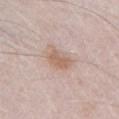Q: Was this lesion biopsied?
A: imaged on a skin check; not biopsied
Q: What are the patient's age and sex?
A: male, in their mid-70s
Q: How was this image acquired?
A: 15 mm crop, total-body photography
Q: Where on the body is the lesion?
A: the chest